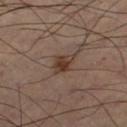No biopsy was performed on this lesion — it was imaged during a full skin examination and was not determined to be concerning.